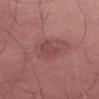workup: imaged on a skin check; not biopsied | subject: male, roughly 50 years of age | tile lighting: white-light illumination | anatomic site: the left thigh | image source: total-body-photography crop, ~15 mm field of view.